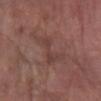Impression:
This lesion was catalogued during total-body skin photography and was not selected for biopsy.
Image and clinical context:
A female patient, roughly 70 years of age. A roughly 15 mm field-of-view crop from a total-body skin photograph. About 5.5 mm across. The lesion is on the right forearm. Imaged with white-light lighting. Automated tile analysis of the lesion measured an area of roughly 8.5 mm² and a shape eccentricity near 0.9. And it measured border irregularity of about 7 on a 0–10 scale, a color-variation rating of about 2/10, and radial color variation of about 0.5.